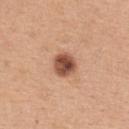| feature | finding |
|---|---|
| follow-up | total-body-photography surveillance lesion; no biopsy |
| automated metrics | an area of roughly 6.5 mm², a shape eccentricity near 0.35, and a shape-asymmetry score of about 0.2 (0 = symmetric); a mean CIELAB color near L≈50 a*≈23 b*≈31 and a normalized border contrast of about 11.5 |
| tile lighting | white-light |
| diameter | about 3 mm |
| image | ~15 mm tile from a whole-body skin photo |
| subject | female, aged around 45 |
| body site | the upper back |